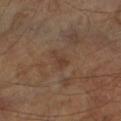{
  "automated_metrics": {
    "area_mm2_approx": 2.5,
    "eccentricity": 0.9,
    "shape_asymmetry": 0.55,
    "cielab_L": 36,
    "cielab_a": 17,
    "cielab_b": 26,
    "vs_skin_darker_L": 6.0,
    "border_irregularity_0_10": 6.0,
    "color_variation_0_10": 0.5,
    "peripheral_color_asymmetry": 0.0,
    "nevus_likeness_0_100": 0
  },
  "patient": {
    "sex": "male",
    "age_approx": 70
  },
  "site": "right forearm",
  "image": {
    "source": "total-body photography crop",
    "field_of_view_mm": 15
  },
  "lighting": "cross-polarized"
}